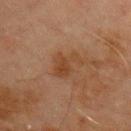Assessment:
Part of a total-body skin-imaging series; this lesion was reviewed on a skin check and was not flagged for biopsy.
Clinical summary:
Captured under cross-polarized illumination. The total-body-photography lesion software estimated about 6 CIELAB-L* units darker than the surrounding skin and a normalized lesion–skin contrast near 6. The software also gave a border-irregularity rating of about 7.5/10 and a within-lesion color-variation index near 2/10. A region of skin cropped from a whole-body photographic capture, roughly 15 mm wide. A male subject aged around 70. From the front of the torso.